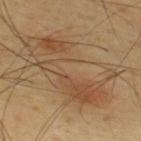{"biopsy_status": "not biopsied; imaged during a skin examination", "site": "back", "patient": {"sex": "male", "age_approx": 65}, "lighting": "cross-polarized", "image": {"source": "total-body photography crop", "field_of_view_mm": 15}, "lesion_size": {"long_diameter_mm_approx": 11.0}}